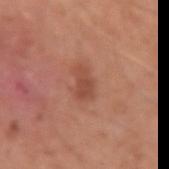Q: Was this lesion biopsied?
A: catalogued during a skin exam; not biopsied
Q: How was this image acquired?
A: total-body-photography crop, ~15 mm field of view
Q: Who is the patient?
A: male, roughly 55 years of age
Q: Where on the body is the lesion?
A: the left upper arm